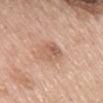workup: no biopsy performed (imaged during a skin exam) | patient: female, approximately 60 years of age | site: the mid back | acquisition: ~15 mm crop, total-body skin-cancer survey.